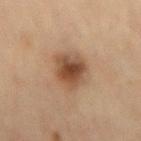Q: Was a biopsy performed?
A: imaged on a skin check; not biopsied
Q: Patient demographics?
A: male, in their mid-40s
Q: What kind of image is this?
A: 15 mm crop, total-body photography
Q: Lesion location?
A: the mid back
Q: What is the lesion's diameter?
A: ~4.5 mm (longest diameter)